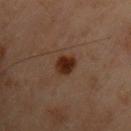The lesion was photographed on a routine skin check and not biopsied; there is no pathology result.
The lesion is located on the chest.
A male subject, approximately 55 years of age.
The lesion's longest dimension is about 3 mm.
The tile uses cross-polarized illumination.
A roughly 15 mm field-of-view crop from a total-body skin photograph.
Automated tile analysis of the lesion measured an average lesion color of about L≈22 a*≈16 b*≈23 (CIELAB), a lesion–skin lightness drop of about 9, and a lesion-to-skin contrast of about 11 (normalized; higher = more distinct). The analysis additionally found lesion-presence confidence of about 100/100.